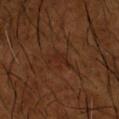A male patient, aged 63–67. On the left forearm. This image is a 15 mm lesion crop taken from a total-body photograph.